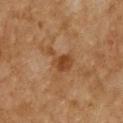Impression:
Part of a total-body skin-imaging series; this lesion was reviewed on a skin check and was not flagged for biopsy.
Background:
The recorded lesion diameter is about 4 mm. The lesion is located on the chest. Automated image analysis of the tile measured an automated nevus-likeness rating near 5 out of 100 and a detector confidence of about 100 out of 100 that the crop contains a lesion. This image is a 15 mm lesion crop taken from a total-body photograph. This is a cross-polarized tile. A female patient about 60 years old.